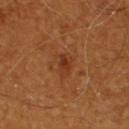Q: Was this lesion biopsied?
A: no biopsy performed (imaged during a skin exam)
Q: Patient demographics?
A: male, approximately 60 years of age
Q: What did automated image analysis measure?
A: a lesion color around L≈32 a*≈24 b*≈33 in CIELAB and a lesion-to-skin contrast of about 6.5 (normalized; higher = more distinct)
Q: What is the anatomic site?
A: the upper back
Q: How was this image acquired?
A: ~15 mm crop, total-body skin-cancer survey